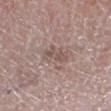Clinical impression: Recorded during total-body skin imaging; not selected for excision or biopsy. Clinical summary: From the leg. Approximately 3.5 mm at its widest. A female patient approximately 65 years of age. Captured under white-light illumination. A close-up tile cropped from a whole-body skin photograph, about 15 mm across. An algorithmic analysis of the crop reported an eccentricity of roughly 0.8 and a shape-asymmetry score of about 0.4 (0 = symmetric). And it measured a color-variation rating of about 2/10 and a peripheral color-asymmetry measure near 0.5.